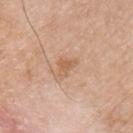follow-up — imaged on a skin check; not biopsied | anatomic site — the chest | imaging modality — ~15 mm tile from a whole-body skin photo | illumination — white-light | patient — male, approximately 55 years of age | lesion diameter — about 2.5 mm.